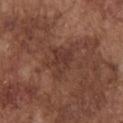Findings:
• site: the chest
• illumination: white-light
• image-analysis metrics: an average lesion color of about L≈35 a*≈20 b*≈24 (CIELAB) and a normalized border contrast of about 6
• subject: male, approximately 75 years of age
• lesion size: ≈4 mm
• image: ~15 mm tile from a whole-body skin photo